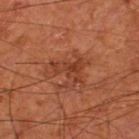workup — no biopsy performed (imaged during a skin exam) | subject — male, approximately 65 years of age | lighting — cross-polarized | lesion diameter — ≈4 mm | site — the left thigh | acquisition — total-body-photography crop, ~15 mm field of view.